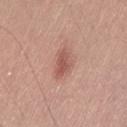| field | value |
|---|---|
| biopsy status | total-body-photography surveillance lesion; no biopsy |
| location | the left thigh |
| patient | male, about 55 years old |
| image | 15 mm crop, total-body photography |
| lesion diameter | about 3.5 mm |
| tile lighting | white-light illumination |